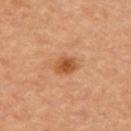Captured during whole-body skin photography for melanoma surveillance; the lesion was not biopsied. An algorithmic analysis of the crop reported roughly 11 lightness units darker than nearby skin. And it measured a border-irregularity index near 2/10, a color-variation rating of about 4/10, and peripheral color asymmetry of about 1. It also reported a detector confidence of about 100 out of 100 that the crop contains a lesion. A male patient, aged approximately 85 or older. On the right upper arm. The recorded lesion diameter is about 3 mm. Captured under cross-polarized illumination. A 15 mm close-up extracted from a 3D total-body photography capture.